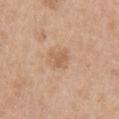Part of a total-body skin-imaging series; this lesion was reviewed on a skin check and was not flagged for biopsy. Approximately 2.5 mm at its widest. The lesion-visualizer software estimated a lesion color around L≈60 a*≈19 b*≈33 in CIELAB, roughly 8 lightness units darker than nearby skin, and a normalized lesion–skin contrast near 5.5. And it measured border irregularity of about 3.5 on a 0–10 scale, internal color variation of about 1.5 on a 0–10 scale, and radial color variation of about 0.5. Captured under white-light illumination. The subject is a male approximately 65 years of age. Cropped from a whole-body photographic skin survey; the tile spans about 15 mm. On the chest.